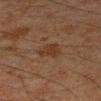{
  "biopsy_status": "not biopsied; imaged during a skin examination",
  "automated_metrics": {
    "area_mm2_approx": 5.0,
    "eccentricity": 0.45,
    "border_irregularity_0_10": 4.0,
    "color_variation_0_10": 2.0,
    "lesion_detection_confidence_0_100": 100
  },
  "lesion_size": {
    "long_diameter_mm_approx": 3.0
  },
  "image": {
    "source": "total-body photography crop",
    "field_of_view_mm": 15
  },
  "site": "right forearm",
  "patient": {
    "sex": "male",
    "age_approx": 45
  },
  "lighting": "cross-polarized"
}